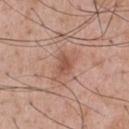biopsy status: total-body-photography surveillance lesion; no biopsy
lesion size: ~3 mm (longest diameter)
lighting: white-light
location: the back
patient: male, in their mid-50s
imaging modality: ~15 mm tile from a whole-body skin photo